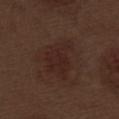{
  "biopsy_status": "not biopsied; imaged during a skin examination",
  "automated_metrics": {
    "color_variation_0_10": 2.0,
    "peripheral_color_asymmetry": 0.5,
    "nevus_likeness_0_100": 5
  },
  "lighting": "white-light",
  "image": {
    "source": "total-body photography crop",
    "field_of_view_mm": 15
  },
  "site": "left thigh",
  "lesion_size": {
    "long_diameter_mm_approx": 5.0
  },
  "patient": {
    "sex": "male",
    "age_approx": 70
  }
}